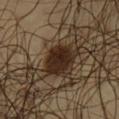automated_metrics:
  cielab_L: 24
  cielab_a: 14
  cielab_b: 23
  vs_skin_darker_L: 13.0
  vs_skin_contrast_norm: 13.5
  nevus_likeness_0_100: 95
  lesion_detection_confidence_0_100: 100
site: front of the torso
image:
  source: total-body photography crop
  field_of_view_mm: 15
patient:
  sex: male
  age_approx: 50
lesion_size:
  long_diameter_mm_approx: 4.0